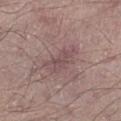workup = catalogued during a skin exam; not biopsied | subject = male, about 60 years old | site = the right lower leg | acquisition = ~15 mm crop, total-body skin-cancer survey | diameter = ≈4.5 mm | lighting = white-light illumination.